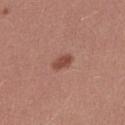- notes · catalogued during a skin exam; not biopsied
- lesion diameter · ~2.5 mm (longest diameter)
- image · ~15 mm tile from a whole-body skin photo
- subject · female, aged around 25
- site · the right thigh
- illumination · white-light illumination